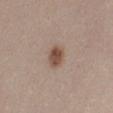No biopsy was performed on this lesion — it was imaged during a full skin examination and was not determined to be concerning.
On the abdomen.
Measured at roughly 3 mm in maximum diameter.
Captured under white-light illumination.
A female subject aged 23 to 27.
A 15 mm close-up extracted from a 3D total-body photography capture.